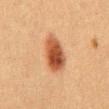Findings:
- notes · catalogued during a skin exam; not biopsied
- lesion size · about 5 mm
- body site · the front of the torso
- lighting · cross-polarized
- acquisition · total-body-photography crop, ~15 mm field of view
- patient · male, aged approximately 40